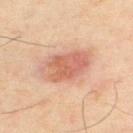Assessment: Imaged during a routine full-body skin examination; the lesion was not biopsied and no histopathology is available. Clinical summary: A male subject in their 60s. A region of skin cropped from a whole-body photographic capture, roughly 15 mm wide. Approximately 5.5 mm at its widest. The lesion is on the upper back. An algorithmic analysis of the crop reported a lesion area of about 16 mm², an eccentricity of roughly 0.75, and a symmetry-axis asymmetry near 0.15. The software also gave a mean CIELAB color near L≈56 a*≈23 b*≈28 and roughly 10 lightness units darker than nearby skin.